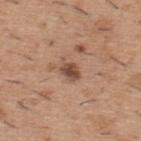– workup · imaged on a skin check; not biopsied
– imaging modality · ~15 mm tile from a whole-body skin photo
– site · the back
– lesion size · ~3 mm (longest diameter)
– subject · male, about 40 years old